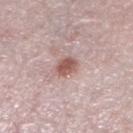Assessment: No biopsy was performed on this lesion — it was imaged during a full skin examination and was not determined to be concerning. Acquisition and patient details: From the left lower leg. This is a white-light tile. Automated tile analysis of the lesion measured a border-irregularity index near 2/10. The software also gave a classifier nevus-likeness of about 90/100 and lesion-presence confidence of about 100/100. The patient is a female approximately 40 years of age. A 15 mm close-up tile from a total-body photography series done for melanoma screening. Measured at roughly 2.5 mm in maximum diameter.